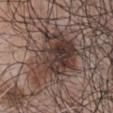biopsy_status: not biopsied; imaged during a skin examination
patient:
  sex: male
  age_approx: 70
site: chest
image:
  source: total-body photography crop
  field_of_view_mm: 15
lighting: white-light